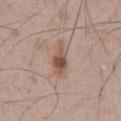This lesion was catalogued during total-body skin photography and was not selected for biopsy. Imaged with white-light lighting. Located on the abdomen. Cropped from a whole-body photographic skin survey; the tile spans about 15 mm. The recorded lesion diameter is about 4.5 mm. A male subject aged 48 to 52.